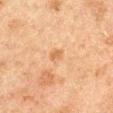No biopsy was performed on this lesion — it was imaged during a full skin examination and was not determined to be concerning. The tile uses cross-polarized illumination. The lesion is on the arm. A region of skin cropped from a whole-body photographic capture, roughly 15 mm wide. Approximately 3 mm at its widest. The patient is a male about 50 years old.